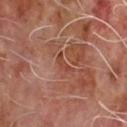Case summary:
– follow-up · total-body-photography surveillance lesion; no biopsy
– image source · ~15 mm tile from a whole-body skin photo
– image-analysis metrics · a footprint of about 2.5 mm², a shape eccentricity near 0.9, and a shape-asymmetry score of about 0.45 (0 = symmetric)
– site · the chest
– lesion size · ~2.5 mm (longest diameter)
– subject · male, aged 63–67
– lighting · cross-polarized illumination